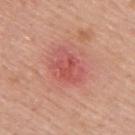{"lighting": "white-light", "patient": {"sex": "male", "age_approx": 50}, "site": "upper back", "image": {"source": "total-body photography crop", "field_of_view_mm": 15}, "automated_metrics": {"border_irregularity_0_10": 3.0, "color_variation_0_10": 4.5, "peripheral_color_asymmetry": 1.5}, "lesion_size": {"long_diameter_mm_approx": 4.5}}